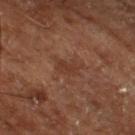Captured during whole-body skin photography for melanoma surveillance; the lesion was not biopsied. The tile uses cross-polarized illumination. A 15 mm close-up extracted from a 3D total-body photography capture. A patient about 65 years old. Longest diameter approximately 3 mm. The lesion-visualizer software estimated an outline eccentricity of about 0.8 (0 = round, 1 = elongated) and a shape-asymmetry score of about 0.35 (0 = symmetric). The analysis additionally found an average lesion color of about L≈36 a*≈21 b*≈28 (CIELAB), about 6 CIELAB-L* units darker than the surrounding skin, and a normalized lesion–skin contrast near 5.5. And it measured border irregularity of about 4.5 on a 0–10 scale and a within-lesion color-variation index near 1/10. And it measured an automated nevus-likeness rating near 0 out of 100 and a detector confidence of about 100 out of 100 that the crop contains a lesion. From the leg.